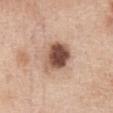Q: Was this lesion biopsied?
A: total-body-photography surveillance lesion; no biopsy
Q: Automated lesion metrics?
A: a classifier nevus-likeness of about 80/100 and a detector confidence of about 100 out of 100 that the crop contains a lesion
Q: How was this image acquired?
A: 15 mm crop, total-body photography
Q: How large is the lesion?
A: ~4.5 mm (longest diameter)
Q: Where on the body is the lesion?
A: the front of the torso
Q: What are the patient's age and sex?
A: female, aged around 40